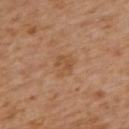follow-up: imaged on a skin check; not biopsied
anatomic site: the back
subject: female, aged approximately 55
acquisition: ~15 mm crop, total-body skin-cancer survey